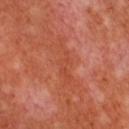follow-up = no biopsy performed (imaged during a skin exam)
lighting = cross-polarized
subject = male, aged approximately 55
body site = the chest
image source = 15 mm crop, total-body photography
lesion diameter = about 5.5 mm